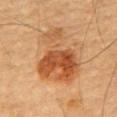biopsy status: no biopsy performed (imaged during a skin exam); diameter: ~8.5 mm (longest diameter); imaging modality: 15 mm crop, total-body photography; tile lighting: cross-polarized illumination; patient: male, aged approximately 85 or older; anatomic site: the front of the torso.